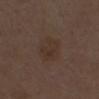The lesion was photographed on a routine skin check and not biopsied; there is no pathology result.
This is a white-light tile.
Measured at roughly 4 mm in maximum diameter.
Cropped from a whole-body photographic skin survey; the tile spans about 15 mm.
A female patient, aged around 50.
The total-body-photography lesion software estimated a within-lesion color-variation index near 2.5/10 and a peripheral color-asymmetry measure near 1.
From the front of the torso.